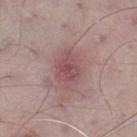The lesion was photographed on a routine skin check and not biopsied; there is no pathology result.
The lesion is on the right thigh.
A male subject aged 68 to 72.
A 15 mm close-up extracted from a 3D total-body photography capture.